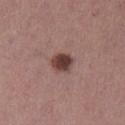Longest diameter approximately 3 mm.
A female subject, in their 60s.
Imaged with white-light lighting.
The total-body-photography lesion software estimated an average lesion color of about L≈41 a*≈20 b*≈21 (CIELAB). The analysis additionally found a within-lesion color-variation index near 4/10 and radial color variation of about 1.
This image is a 15 mm lesion crop taken from a total-body photograph.
From the leg.